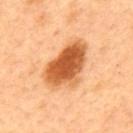Approximately 6.5 mm at its widest.
The lesion is on the mid back.
A male patient in their 50s.
A lesion tile, about 15 mm wide, cut from a 3D total-body photograph.